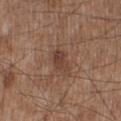Q: Was a biopsy performed?
A: no biopsy performed (imaged during a skin exam)
Q: Illumination type?
A: white-light
Q: Where on the body is the lesion?
A: the right lower leg
Q: What is the lesion's diameter?
A: ~3 mm (longest diameter)
Q: What kind of image is this?
A: ~15 mm crop, total-body skin-cancer survey
Q: What are the patient's age and sex?
A: male, aged around 55